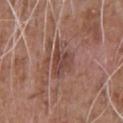Q: Was a biopsy performed?
A: imaged on a skin check; not biopsied
Q: What is the anatomic site?
A: the upper back
Q: Automated lesion metrics?
A: a lesion area of about 7 mm², a shape eccentricity near 0.7, and two-axis asymmetry of about 0.45; an automated nevus-likeness rating near 0 out of 100
Q: How large is the lesion?
A: ≈4 mm
Q: How was this image acquired?
A: total-body-photography crop, ~15 mm field of view
Q: Who is the patient?
A: male, aged 58–62